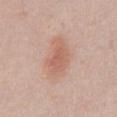workup: catalogued during a skin exam; not biopsied
patient: male, approximately 40 years of age
tile lighting: white-light illumination
site: the abdomen
image source: 15 mm crop, total-body photography